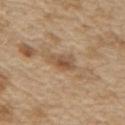The lesion was photographed on a routine skin check and not biopsied; there is no pathology result.
Automated tile analysis of the lesion measured a footprint of about 5.5 mm² and an eccentricity of roughly 0.75. The software also gave a classifier nevus-likeness of about 20/100 and a lesion-detection confidence of about 100/100.
The lesion is located on the right upper arm.
The subject is a male roughly 70 years of age.
The recorded lesion diameter is about 3 mm.
A region of skin cropped from a whole-body photographic capture, roughly 15 mm wide.
The tile uses white-light illumination.